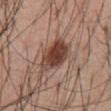follow-up: no biopsy performed (imaged during a skin exam)
illumination: white-light illumination
subject: male, aged approximately 55
image: 15 mm crop, total-body photography
automated lesion analysis: a lesion–skin lightness drop of about 14 and a normalized lesion–skin contrast near 11; an automated nevus-likeness rating near 95 out of 100 and a lesion-detection confidence of about 100/100
location: the abdomen
size: ~5 mm (longest diameter)